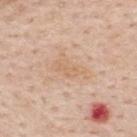Captured during whole-body skin photography for melanoma surveillance; the lesion was not biopsied. Automated image analysis of the tile measured an eccentricity of roughly 0.85 and a shape-asymmetry score of about 0.45 (0 = symmetric). The analysis additionally found a border-irregularity rating of about 5.5/10, a within-lesion color-variation index near 1.5/10, and radial color variation of about 0.5. The lesion is on the back. A 15 mm close-up tile from a total-body photography series done for melanoma screening. A male subject, aged 58–62. This is a white-light tile.